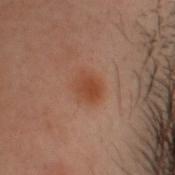{
  "lighting": "cross-polarized",
  "site": "head or neck",
  "image": {
    "source": "total-body photography crop",
    "field_of_view_mm": 15
  },
  "lesion_size": {
    "long_diameter_mm_approx": 3.0
  },
  "automated_metrics": {
    "border_irregularity_0_10": 1.5,
    "color_variation_0_10": 2.5,
    "peripheral_color_asymmetry": 1.0,
    "nevus_likeness_0_100": 95
  },
  "patient": {
    "sex": "male",
    "age_approx": 30
  }
}